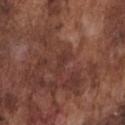The lesion was photographed on a routine skin check and not biopsied; there is no pathology result.
The patient is a male aged approximately 75.
The lesion is located on the chest.
A 15 mm close-up tile from a total-body photography series done for melanoma screening.